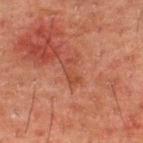Part of a total-body skin-imaging series; this lesion was reviewed on a skin check and was not flagged for biopsy.
The lesion is located on the upper back.
The total-body-photography lesion software estimated a lesion area of about 4 mm², an outline eccentricity of about 0.85 (0 = round, 1 = elongated), and a shape-asymmetry score of about 0.45 (0 = symmetric). The software also gave a border-irregularity index near 6.5/10, a within-lesion color-variation index near 0.5/10, and peripheral color asymmetry of about 0. And it measured an automated nevus-likeness rating near 0 out of 100 and a detector confidence of about 100 out of 100 that the crop contains a lesion.
Approximately 3 mm at its widest.
A lesion tile, about 15 mm wide, cut from a 3D total-body photograph.
The patient is a male aged around 50.